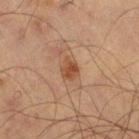biopsy_status: not biopsied; imaged during a skin examination
image:
  source: total-body photography crop
  field_of_view_mm: 15
site: left thigh
automated_metrics:
  cielab_L: 38
  cielab_a: 18
  cielab_b: 28
  vs_skin_darker_L: 8.0
  color_variation_0_10: 2.5
patient:
  sex: male
  age_approx: 65
lighting: cross-polarized